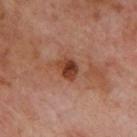No biopsy was performed on this lesion — it was imaged during a full skin examination and was not determined to be concerning.
This is a cross-polarized tile.
A 15 mm crop from a total-body photograph taken for skin-cancer surveillance.
A male patient, aged approximately 70.
Measured at roughly 2.5 mm in maximum diameter.
Automated image analysis of the tile measured an average lesion color of about L≈39 a*≈25 b*≈31 (CIELAB), roughly 12 lightness units darker than nearby skin, and a normalized border contrast of about 10.
On the upper back.